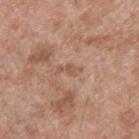Part of a total-body skin-imaging series; this lesion was reviewed on a skin check and was not flagged for biopsy.
Automated image analysis of the tile measured a lesion area of about 3 mm² and a shape eccentricity near 0.9.
Approximately 2.5 mm at its widest.
A male subject, in their mid-50s.
A 15 mm crop from a total-body photograph taken for skin-cancer surveillance.
The tile uses white-light illumination.
The lesion is on the upper back.